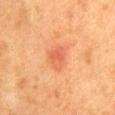This lesion was catalogued during total-body skin photography and was not selected for biopsy.
The recorded lesion diameter is about 3 mm.
A region of skin cropped from a whole-body photographic capture, roughly 15 mm wide.
From the chest.
The subject is a female roughly 50 years of age.